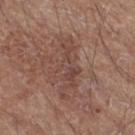Part of a total-body skin-imaging series; this lesion was reviewed on a skin check and was not flagged for biopsy.
Measured at roughly 7 mm in maximum diameter.
On the right thigh.
Automated image analysis of the tile measured two-axis asymmetry of about 0.45. The analysis additionally found about 7 CIELAB-L* units darker than the surrounding skin and a normalized border contrast of about 5.5.
A 15 mm crop from a total-body photograph taken for skin-cancer surveillance.
The tile uses white-light illumination.
The patient is a male about 65 years old.